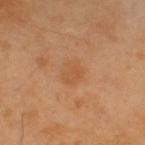<lesion>
  <biopsy_status>not biopsied; imaged during a skin examination</biopsy_status>
  <patient>
    <sex>male</sex>
    <age_approx>40</age_approx>
  </patient>
  <image>
    <source>total-body photography crop</source>
    <field_of_view_mm>15</field_of_view_mm>
  </image>
  <site>upper back</site>
</lesion>